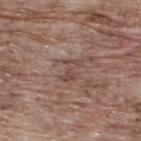* notes: no biopsy performed (imaged during a skin exam)
* patient: male, approximately 70 years of age
* body site: the upper back
* image source: ~15 mm tile from a whole-body skin photo